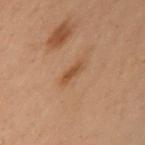biopsy status: catalogued during a skin exam; not biopsied | automated metrics: a footprint of about 2.5 mm² and an eccentricity of roughly 0.95; a lesion color around L≈41 a*≈19 b*≈31 in CIELAB and a normalized border contrast of about 7; a border-irregularity rating of about 3/10, a within-lesion color-variation index near 0/10, and radial color variation of about 0; an automated nevus-likeness rating near 40 out of 100 and a lesion-detection confidence of about 100/100 | diameter: ~2.5 mm (longest diameter) | illumination: cross-polarized | site: the left upper arm | subject: female, aged 53 to 57 | imaging modality: ~15 mm tile from a whole-body skin photo.